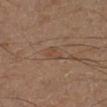follow-up = catalogued during a skin exam; not biopsied | image-analysis metrics = a lesion area of about 4 mm² | subject = male, roughly 60 years of age | diameter = ~2.5 mm (longest diameter) | acquisition = total-body-photography crop, ~15 mm field of view | illumination = cross-polarized illumination | anatomic site = the right lower leg.